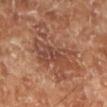workup: no biopsy performed (imaged during a skin exam) | image source: ~15 mm crop, total-body skin-cancer survey | subject: male, in their 70s | body site: the left lower leg | tile lighting: cross-polarized illumination | lesion size: about 7.5 mm.